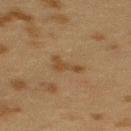workup = catalogued during a skin exam; not biopsied | image = ~15 mm crop, total-body skin-cancer survey | patient = female, approximately 40 years of age | body site = the upper back | lesion diameter = about 3 mm.